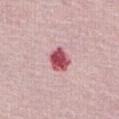workup — total-body-photography surveillance lesion; no biopsy | subject — female, about 65 years old | image-analysis metrics — an area of roughly 6.5 mm², an eccentricity of roughly 0.5, and a shape-asymmetry score of about 0.2 (0 = symmetric); border irregularity of about 2 on a 0–10 scale and peripheral color asymmetry of about 1.5; a nevus-likeness score of about 0/100 and a lesion-detection confidence of about 100/100 | lesion diameter — about 3 mm | image — ~15 mm crop, total-body skin-cancer survey | body site — the front of the torso | illumination — white-light.